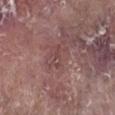| field | value |
|---|---|
| workup | catalogued during a skin exam; not biopsied |
| acquisition | 15 mm crop, total-body photography |
| subject | female, in their mid-70s |
| diameter | about 2.5 mm |
| lighting | white-light |
| anatomic site | the right lower leg |